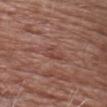<case>
<biopsy_status>not biopsied; imaged during a skin examination</biopsy_status>
<lesion_size>
  <long_diameter_mm_approx>2.5</long_diameter_mm_approx>
</lesion_size>
<image>
  <source>total-body photography crop</source>
  <field_of_view_mm>15</field_of_view_mm>
</image>
<patient>
  <sex>male</sex>
  <age_approx>80</age_approx>
</patient>
<site>head or neck</site>
</case>